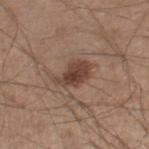subject = male, in their mid- to late 40s; image = 15 mm crop, total-body photography; illumination = white-light; body site = the right lower leg; size = ~4 mm (longest diameter).